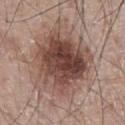biopsy status = no biopsy performed (imaged during a skin exam)
site = the abdomen
lesion diameter = about 7 mm
subject = male, aged approximately 65
lighting = white-light illumination
image = 15 mm crop, total-body photography
TBP lesion metrics = an area of roughly 34 mm², an eccentricity of roughly 0.4, and two-axis asymmetry of about 0.25; a classifier nevus-likeness of about 65/100